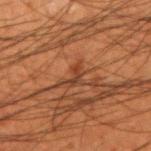Impression:
This lesion was catalogued during total-body skin photography and was not selected for biopsy.